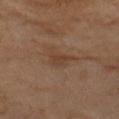| field | value |
|---|---|
| follow-up | total-body-photography surveillance lesion; no biopsy |